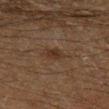Case summary:
- notes: catalogued during a skin exam; not biopsied
- subject: male, roughly 60 years of age
- imaging modality: ~15 mm crop, total-body skin-cancer survey
- diameter: about 3 mm
- site: the left lower leg
- tile lighting: cross-polarized illumination